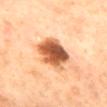biopsy_status: not biopsied; imaged during a skin examination
site: back
image:
  source: total-body photography crop
  field_of_view_mm: 15
lighting: cross-polarized
patient:
  sex: female
  age_approx: 65
automated_metrics:
  area_mm2_approx: 15.0
  eccentricity: 0.4
  shape_asymmetry: 0.2
  nevus_likeness_0_100: 75
lesion_size:
  long_diameter_mm_approx: 4.5